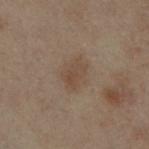Part of a total-body skin-imaging series; this lesion was reviewed on a skin check and was not flagged for biopsy.
An algorithmic analysis of the crop reported an area of roughly 5.5 mm², an outline eccentricity of about 0.75 (0 = round, 1 = elongated), and a shape-asymmetry score of about 0.2 (0 = symmetric). The software also gave an average lesion color of about L≈37 a*≈12 b*≈22 (CIELAB), a lesion–skin lightness drop of about 5, and a lesion-to-skin contrast of about 5.5 (normalized; higher = more distinct).
On the leg.
The recorded lesion diameter is about 3 mm.
The patient is a female aged approximately 55.
A lesion tile, about 15 mm wide, cut from a 3D total-body photograph.
Imaged with cross-polarized lighting.